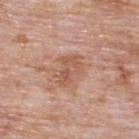Notes:
* follow-up — catalogued during a skin exam; not biopsied
* subject — male, aged 73–77
* anatomic site — the upper back
* image source — ~15 mm tile from a whole-body skin photo
* diameter — about 3.5 mm